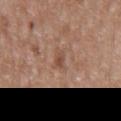Findings:
• biopsy status · catalogued during a skin exam; not biopsied
• subject · male, about 50 years old
• image-analysis metrics · an area of roughly 2.5 mm², an outline eccentricity of about 0.9 (0 = round, 1 = elongated), and a shape-asymmetry score of about 0.3 (0 = symmetric); an average lesion color of about L≈48 a*≈20 b*≈28 (CIELAB); an automated nevus-likeness rating near 0 out of 100
• body site · the mid back
• lesion diameter · ~2.5 mm (longest diameter)
• image · ~15 mm crop, total-body skin-cancer survey